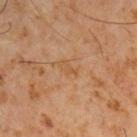This lesion was catalogued during total-body skin photography and was not selected for biopsy. This image is a 15 mm lesion crop taken from a total-body photograph. On the right upper arm. A male patient, roughly 60 years of age.